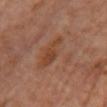The lesion was photographed on a routine skin check and not biopsied; there is no pathology result. Automated tile analysis of the lesion measured a lesion color around L≈46 a*≈21 b*≈31 in CIELAB, a lesion–skin lightness drop of about 5, and a normalized border contrast of about 5. And it measured a color-variation rating of about 5/10 and radial color variation of about 1.5. It also reported a classifier nevus-likeness of about 5/100 and a lesion-detection confidence of about 100/100. A female patient about 60 years old. A region of skin cropped from a whole-body photographic capture, roughly 15 mm wide. On the chest. This is a cross-polarized tile.